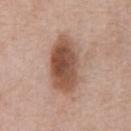biopsy status — no biopsy performed (imaged during a skin exam)
automated metrics — a border-irregularity rating of about 2/10
body site — the chest
image — 15 mm crop, total-body photography
subject — male, aged approximately 75
illumination — white-light
size — ≈7 mm